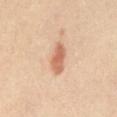The tile uses cross-polarized illumination.
The lesion is located on the abdomen.
The recorded lesion diameter is about 4 mm.
This image is a 15 mm lesion crop taken from a total-body photograph.
A female patient, aged around 40.